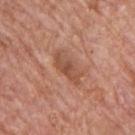Notes:
- notes · no biopsy performed (imaged during a skin exam)